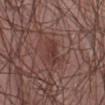The lesion was photographed on a routine skin check and not biopsied; there is no pathology result. Longest diameter approximately 3 mm. This is a white-light tile. The subject is a male aged around 55. From the front of the torso. This image is a 15 mm lesion crop taken from a total-body photograph.